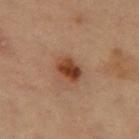{
  "biopsy_status": "not biopsied; imaged during a skin examination",
  "lighting": "cross-polarized",
  "lesion_size": {
    "long_diameter_mm_approx": 3.0
  },
  "patient": {
    "sex": "female",
    "age_approx": 70
  },
  "site": "leg",
  "image": {
    "source": "total-body photography crop",
    "field_of_view_mm": 15
  }
}